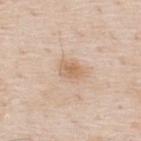The lesion was photographed on a routine skin check and not biopsied; there is no pathology result. A male patient aged around 80. From the upper back. Imaged with white-light lighting. Approximately 2.5 mm at its widest. A close-up tile cropped from a whole-body skin photograph, about 15 mm across.